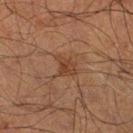Assessment: The lesion was photographed on a routine skin check and not biopsied; there is no pathology result. Context: Located on the right lower leg. Captured under cross-polarized illumination. A close-up tile cropped from a whole-body skin photograph, about 15 mm across. A male patient approximately 70 years of age.